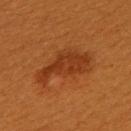biopsy status: imaged on a skin check; not biopsied | acquisition: total-body-photography crop, ~15 mm field of view | diameter: ~7 mm (longest diameter) | subject: female, aged around 30 | site: the upper back | lighting: cross-polarized.